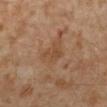The lesion was tiled from a total-body skin photograph and was not biopsied. On the leg. Automated image analysis of the tile measured an area of roughly 7.5 mm², a shape eccentricity near 0.75, and two-axis asymmetry of about 0.35. And it measured a lesion–skin lightness drop of about 6 and a normalized lesion–skin contrast near 5.5. The analysis additionally found border irregularity of about 3.5 on a 0–10 scale. A region of skin cropped from a whole-body photographic capture, roughly 15 mm wide. The recorded lesion diameter is about 4 mm. A male subject aged 28–32.